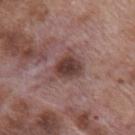Q: What kind of image is this?
A: total-body-photography crop, ~15 mm field of view
Q: What are the patient's age and sex?
A: male, about 70 years old
Q: Where on the body is the lesion?
A: the mid back